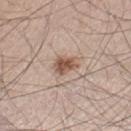Recorded during total-body skin imaging; not selected for excision or biopsy. A 15 mm crop from a total-body photograph taken for skin-cancer surveillance. Located on the left thigh. The lesion's longest dimension is about 3 mm. A male subject, in their mid- to late 40s.